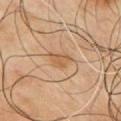biopsy status: no biopsy performed (imaged during a skin exam); body site: the chest; patient: male, aged around 65; lighting: cross-polarized illumination; imaging modality: ~15 mm tile from a whole-body skin photo.